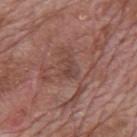Findings:
- notes: no biopsy performed (imaged during a skin exam)
- subject: male, aged around 70
- lesion size: ≈2.5 mm
- location: the mid back
- tile lighting: white-light illumination
- image: total-body-photography crop, ~15 mm field of view